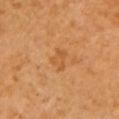No biopsy was performed on this lesion — it was imaged during a full skin examination and was not determined to be concerning.
The patient is a female approximately 55 years of age.
Cropped from a total-body skin-imaging series; the visible field is about 15 mm.
From the left upper arm.